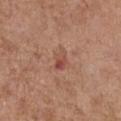workup=no biopsy performed (imaged during a skin exam)
tile lighting=white-light
location=the front of the torso
subject=female, aged around 75
image source=total-body-photography crop, ~15 mm field of view